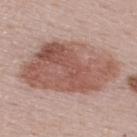Impression:
The lesion was tiled from a total-body skin photograph and was not biopsied.
Clinical summary:
The lesion is located on the upper back. The tile uses white-light illumination. Cropped from a whole-body photographic skin survey; the tile spans about 15 mm. A male patient aged 58 to 62. The lesion-visualizer software estimated a footprint of about 50 mm² and an outline eccentricity of about 0.8 (0 = round, 1 = elongated).